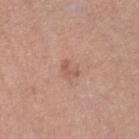Clinical impression: The lesion was photographed on a routine skin check and not biopsied; there is no pathology result. Image and clinical context: The subject is a female in their mid-50s. Cropped from a whole-body photographic skin survey; the tile spans about 15 mm. Captured under white-light illumination. On the right lower leg.